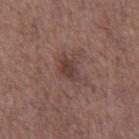Q: What is the anatomic site?
A: the abdomen
Q: What is the imaging modality?
A: ~15 mm tile from a whole-body skin photo
Q: Who is the patient?
A: male, about 70 years old